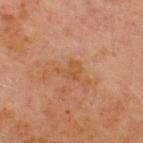This lesion was catalogued during total-body skin photography and was not selected for biopsy. A male patient, roughly 70 years of age. The lesion's longest dimension is about 3 mm. Located on the chest. A region of skin cropped from a whole-body photographic capture, roughly 15 mm wide.